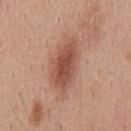The lesion was tiled from a total-body skin photograph and was not biopsied. About 6.5 mm across. Captured under white-light illumination. Cropped from a total-body skin-imaging series; the visible field is about 15 mm. The lesion-visualizer software estimated a lesion area of about 15 mm², an outline eccentricity of about 0.9 (0 = round, 1 = elongated), and a shape-asymmetry score of about 0.25 (0 = symmetric). The lesion is located on the mid back. A male patient about 40 years old.